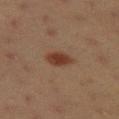Q: Was a biopsy performed?
A: total-body-photography surveillance lesion; no biopsy
Q: What kind of image is this?
A: 15 mm crop, total-body photography
Q: What is the anatomic site?
A: the leg
Q: Patient demographics?
A: female, aged around 20
Q: What did automated image analysis measure?
A: a symmetry-axis asymmetry near 0.15; an average lesion color of about L≈32 a*≈20 b*≈25 (CIELAB) and roughly 10 lightness units darker than nearby skin
Q: Illumination type?
A: cross-polarized illumination
Q: How large is the lesion?
A: ≈3 mm